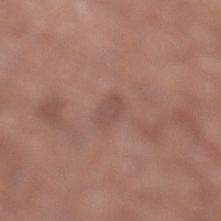No biopsy was performed on this lesion — it was imaged during a full skin examination and was not determined to be concerning. The lesion's longest dimension is about 2.5 mm. The patient is a female aged 78 to 82. Imaged with white-light lighting. This image is a 15 mm lesion crop taken from a total-body photograph. On the leg.